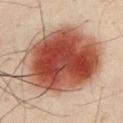Clinical impression: The lesion was tiled from a total-body skin photograph and was not biopsied. Background: A male subject, aged approximately 50. Approximately 11.5 mm at its widest. Automated tile analysis of the lesion measured an outline eccentricity of about 0.65 (0 = round, 1 = elongated) and two-axis asymmetry of about 0.1. Located on the chest. The tile uses cross-polarized illumination. This image is a 15 mm lesion crop taken from a total-body photograph.